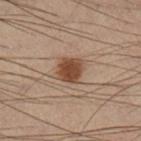| key | value |
|---|---|
| subject | male, aged 53 to 57 |
| illumination | cross-polarized illumination |
| size | ≈3 mm |
| body site | the right lower leg |
| image source | 15 mm crop, total-body photography |